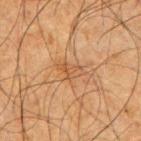The lesion was photographed on a routine skin check and not biopsied; there is no pathology result. The lesion is located on the right upper arm. This image is a 15 mm lesion crop taken from a total-body photograph. A male patient aged around 65. The tile uses cross-polarized illumination. The lesion's longest dimension is about 3 mm.